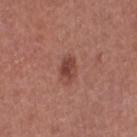Image and clinical context: Captured under white-light illumination. Approximately 3 mm at its widest. Located on the leg. Automated tile analysis of the lesion measured an average lesion color of about L≈43 a*≈25 b*≈25 (CIELAB), a lesion–skin lightness drop of about 11, and a lesion-to-skin contrast of about 8 (normalized; higher = more distinct). The analysis additionally found an automated nevus-likeness rating near 80 out of 100 and lesion-presence confidence of about 100/100. A female patient aged 48 to 52. A region of skin cropped from a whole-body photographic capture, roughly 15 mm wide.